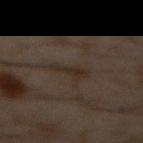| field | value |
|---|---|
| follow-up | catalogued during a skin exam; not biopsied |
| subject | male, in their 60s |
| TBP lesion metrics | a footprint of about 4 mm², an outline eccentricity of about 0.9 (0 = round, 1 = elongated), and two-axis asymmetry of about 0.45; a lesion color around L≈24 a*≈10 b*≈18 in CIELAB and a normalized border contrast of about 6.5; border irregularity of about 6 on a 0–10 scale and internal color variation of about 0.5 on a 0–10 scale; a classifier nevus-likeness of about 0/100 and a detector confidence of about 75 out of 100 that the crop contains a lesion |
| illumination | cross-polarized |
| image source | 15 mm crop, total-body photography |
| anatomic site | the abdomen |